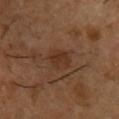No biopsy was performed on this lesion — it was imaged during a full skin examination and was not determined to be concerning. The lesion is located on the right upper arm. Approximately 2.5 mm at its widest. The patient is a male in their mid- to late 50s. A 15 mm crop from a total-body photograph taken for skin-cancer surveillance.